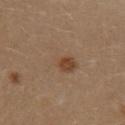* workup · catalogued during a skin exam; not biopsied
* diameter · ~4 mm (longest diameter)
* anatomic site · the left upper arm
* subject · female, aged around 30
* illumination · cross-polarized illumination
* image · 15 mm crop, total-body photography